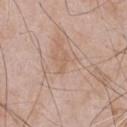Part of a total-body skin-imaging series; this lesion was reviewed on a skin check and was not flagged for biopsy. Imaged with white-light lighting. A male subject approximately 50 years of age. From the chest. A roughly 15 mm field-of-view crop from a total-body skin photograph. Measured at roughly 2.5 mm in maximum diameter.